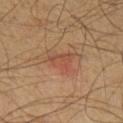Impression:
The lesion was photographed on a routine skin check and not biopsied; there is no pathology result.
Context:
Imaged with cross-polarized lighting. An algorithmic analysis of the crop reported a nevus-likeness score of about 0/100 and lesion-presence confidence of about 100/100. The lesion is on the right lower leg. The patient is a male aged 53 to 57. Approximately 3.5 mm at its widest. A 15 mm crop from a total-body photograph taken for skin-cancer surveillance.